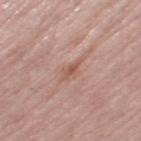<record>
<biopsy_status>not biopsied; imaged during a skin examination</biopsy_status>
<patient>
  <sex>female</sex>
  <age_approx>65</age_approx>
</patient>
<site>leg</site>
<lighting>white-light</lighting>
<image>
  <source>total-body photography crop</source>
  <field_of_view_mm>15</field_of_view_mm>
</image>
<lesion_size>
  <long_diameter_mm_approx>2.5</long_diameter_mm_approx>
</lesion_size>
</record>